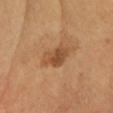| key | value |
|---|---|
| workup | total-body-photography surveillance lesion; no biopsy |
| acquisition | ~15 mm tile from a whole-body skin photo |
| body site | the head or neck |
| patient | male, aged 53 to 57 |